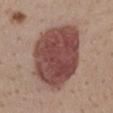Part of a total-body skin-imaging series; this lesion was reviewed on a skin check and was not flagged for biopsy.